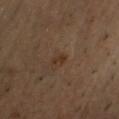This image is a 15 mm lesion crop taken from a total-body photograph. A male subject, aged 48–52. The lesion is located on the chest. The total-body-photography lesion software estimated two-axis asymmetry of about 0.4. This is a cross-polarized tile.